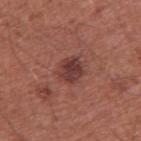<lesion>
  <biopsy_status>not biopsied; imaged during a skin examination</biopsy_status>
  <image>
    <source>total-body photography crop</source>
    <field_of_view_mm>15</field_of_view_mm>
  </image>
  <site>right upper arm</site>
  <lighting>white-light</lighting>
  <patient>
    <sex>male</sex>
    <age_approx>60</age_approx>
  </patient>
  <lesion_size>
    <long_diameter_mm_approx>3.0</long_diameter_mm_approx>
  </lesion_size>
  <automated_metrics>
    <area_mm2_approx>7.0</area_mm2_approx>
    <shape_asymmetry>0.2</shape_asymmetry>
    <nevus_likeness_0_100>65</nevus_likeness_0_100>
    <lesion_detection_confidence_0_100>100</lesion_detection_confidence_0_100>
  </automated_metrics>
</lesion>